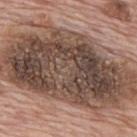Impression:
Recorded during total-body skin imaging; not selected for excision or biopsy.
Image and clinical context:
The tile uses white-light illumination. The total-body-photography lesion software estimated an average lesion color of about L≈47 a*≈16 b*≈24 (CIELAB), roughly 16 lightness units darker than nearby skin, and a lesion-to-skin contrast of about 11 (normalized; higher = more distinct). And it measured a border-irregularity index near 4/10 and radial color variation of about 3.5. The software also gave a classifier nevus-likeness of about 0/100 and a detector confidence of about 95 out of 100 that the crop contains a lesion. A male subject, about 70 years old. The lesion is on the upper back. A 15 mm close-up tile from a total-body photography series done for melanoma screening.